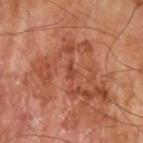  biopsy_status: not biopsied; imaged during a skin examination
  lesion_size:
    long_diameter_mm_approx: 1.5
  lighting: cross-polarized
  site: left upper arm
  patient:
    sex: male
    age_approx: 65
  image:
    source: total-body photography crop
    field_of_view_mm: 15